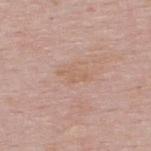The subject is a male aged around 50. The tile uses white-light illumination. A roughly 15 mm field-of-view crop from a total-body skin photograph. Automated tile analysis of the lesion measured an area of roughly 4 mm² and an outline eccentricity of about 0.9 (0 = round, 1 = elongated). And it measured border irregularity of about 4 on a 0–10 scale, a color-variation rating of about 0.5/10, and peripheral color asymmetry of about 0.5. The lesion is on the back.